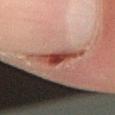Part of a total-body skin-imaging series; this lesion was reviewed on a skin check and was not flagged for biopsy. A 15 mm crop from a total-body photograph taken for skin-cancer surveillance. From the arm. Measured at roughly 5.5 mm in maximum diameter. Captured under cross-polarized illumination. A male subject aged approximately 50.